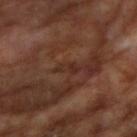Imaged during a routine full-body skin examination; the lesion was not biopsied and no histopathology is available. The lesion is on the right upper arm. Cropped from a whole-body photographic skin survey; the tile spans about 15 mm. The patient is a male about 65 years old.